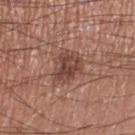| feature | finding |
|---|---|
| follow-up | total-body-photography surveillance lesion; no biopsy |
| subject | male, aged around 55 |
| body site | the left thigh |
| image source | ~15 mm crop, total-body skin-cancer survey |